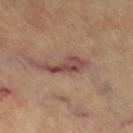Imaged during a routine full-body skin examination; the lesion was not biopsied and no histopathology is available.
The patient is a female aged approximately 60.
Automated image analysis of the tile measured a lesion area of about 6 mm², an eccentricity of roughly 0.95, and two-axis asymmetry of about 0.5. It also reported a lesion color around L≈45 a*≈23 b*≈23 in CIELAB and a normalized lesion–skin contrast near 9.5. It also reported a border-irregularity rating of about 6/10, a within-lesion color-variation index near 4/10, and a peripheral color-asymmetry measure near 1.5.
A roughly 15 mm field-of-view crop from a total-body skin photograph.
Longest diameter approximately 5 mm.
The lesion is on the left leg.